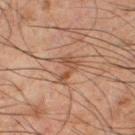Recorded during total-body skin imaging; not selected for excision or biopsy. Approximately 3.5 mm at its widest. This is a cross-polarized tile. Automated tile analysis of the lesion measured a mean CIELAB color near L≈41 a*≈18 b*≈26, roughly 8 lightness units darker than nearby skin, and a normalized border contrast of about 7. And it measured border irregularity of about 7 on a 0–10 scale, a color-variation rating of about 2/10, and a peripheral color-asymmetry measure near 1. The analysis additionally found an automated nevus-likeness rating near 0 out of 100 and lesion-presence confidence of about 100/100. The lesion is located on the right thigh. A roughly 15 mm field-of-view crop from a total-body skin photograph. The subject is a male in their mid- to late 60s.